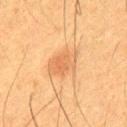lighting=cross-polarized illumination
diameter=about 4.5 mm
imaging modality=total-body-photography crop, ~15 mm field of view
anatomic site=the upper back
patient=male, roughly 35 years of age